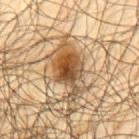{
  "biopsy_status": "not biopsied; imaged during a skin examination",
  "lesion_size": {
    "long_diameter_mm_approx": 7.5
  },
  "site": "upper back",
  "image": {
    "source": "total-body photography crop",
    "field_of_view_mm": 15
  },
  "automated_metrics": {
    "border_irregularity_0_10": 5.5,
    "color_variation_0_10": 9.5,
    "peripheral_color_asymmetry": 3.0,
    "nevus_likeness_0_100": 95,
    "lesion_detection_confidence_0_100": 100
  },
  "patient": {
    "sex": "male",
    "age_approx": 65
  },
  "lighting": "cross-polarized"
}